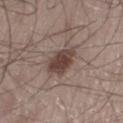No biopsy was performed on this lesion — it was imaged during a full skin examination and was not determined to be concerning. A male patient, about 55 years old. Imaged with white-light lighting. Measured at roughly 3.5 mm in maximum diameter. On the left thigh. An algorithmic analysis of the crop reported a mean CIELAB color near L≈42 a*≈16 b*≈21. And it measured a color-variation rating of about 3.5/10 and a peripheral color-asymmetry measure near 1. The software also gave a nevus-likeness score of about 95/100 and a lesion-detection confidence of about 100/100. A close-up tile cropped from a whole-body skin photograph, about 15 mm across.